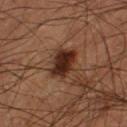Q: Was this lesion biopsied?
A: no biopsy performed (imaged during a skin exam)
Q: Lesion location?
A: the left thigh
Q: What are the patient's age and sex?
A: male, about 60 years old
Q: What is the imaging modality?
A: ~15 mm crop, total-body skin-cancer survey